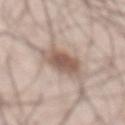Q: Was a biopsy performed?
A: imaged on a skin check; not biopsied
Q: What kind of image is this?
A: ~15 mm tile from a whole-body skin photo
Q: What lighting was used for the tile?
A: white-light illumination
Q: Patient demographics?
A: male, in their mid- to late 50s
Q: How large is the lesion?
A: ~4.5 mm (longest diameter)
Q: What is the anatomic site?
A: the front of the torso
Q: Automated lesion metrics?
A: an outline eccentricity of about 0.55 (0 = round, 1 = elongated) and a shape-asymmetry score of about 0.2 (0 = symmetric); about 13 CIELAB-L* units darker than the surrounding skin and a normalized lesion–skin contrast near 8.5; a border-irregularity index near 2/10